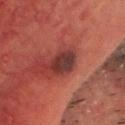<tbp_lesion>
  <biopsy_status>not biopsied; imaged during a skin examination</biopsy_status>
  <patient>
    <sex>male</sex>
    <age_approx>75</age_approx>
  </patient>
  <lesion_size>
    <long_diameter_mm_approx>3.0</long_diameter_mm_approx>
  </lesion_size>
  <image>
    <source>total-body photography crop</source>
    <field_of_view_mm>15</field_of_view_mm>
  </image>
  <site>head or neck</site>
</tbp_lesion>